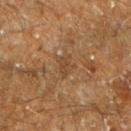Recorded during total-body skin imaging; not selected for excision or biopsy.
Located on the left lower leg.
Approximately 2.5 mm at its widest.
Cropped from a whole-body photographic skin survey; the tile spans about 15 mm.
The subject is a male aged around 60.
Automated image analysis of the tile measured a footprint of about 4 mm², an outline eccentricity of about 0.6 (0 = round, 1 = elongated), and two-axis asymmetry of about 0.4. The analysis additionally found a mean CIELAB color near L≈33 a*≈14 b*≈27, about 5 CIELAB-L* units darker than the surrounding skin, and a normalized border contrast of about 5. And it measured a border-irregularity index near 4/10, a color-variation rating of about 1.5/10, and radial color variation of about 0.5.